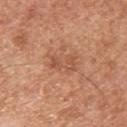{"biopsy_status": "not biopsied; imaged during a skin examination", "lighting": "white-light", "image": {"source": "total-body photography crop", "field_of_view_mm": 15}, "automated_metrics": {"area_mm2_approx": 5.5, "eccentricity": 0.85, "shape_asymmetry": 0.25, "cielab_L": 54, "cielab_a": 25, "cielab_b": 33, "vs_skin_darker_L": 8.0, "vs_skin_contrast_norm": 5.5, "border_irregularity_0_10": 4.5, "color_variation_0_10": 2.5, "peripheral_color_asymmetry": 1.0}, "site": "upper back", "lesion_size": {"long_diameter_mm_approx": 3.5}, "patient": {"sex": "male", "age_approx": 30}}